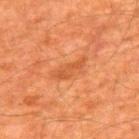Recorded during total-body skin imaging; not selected for excision or biopsy. An algorithmic analysis of the crop reported a lesion color around L≈43 a*≈25 b*≈35 in CIELAB and a lesion–skin lightness drop of about 6. The software also gave a peripheral color-asymmetry measure near 0.5. This is a cross-polarized tile. A male subject, about 60 years old. A close-up tile cropped from a whole-body skin photograph, about 15 mm across. The lesion's longest dimension is about 4.5 mm. The lesion is located on the upper back.